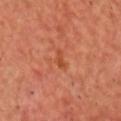This lesion was catalogued during total-body skin photography and was not selected for biopsy.